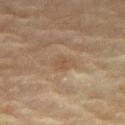Recorded during total-body skin imaging; not selected for excision or biopsy.
Imaged with cross-polarized lighting.
Located on the right thigh.
A female patient, aged 78 to 82.
Measured at roughly 3 mm in maximum diameter.
Cropped from a total-body skin-imaging series; the visible field is about 15 mm.
Automated tile analysis of the lesion measured a lesion area of about 4 mm², a shape eccentricity near 0.75, and a symmetry-axis asymmetry near 0.25. And it measured a border-irregularity rating of about 2.5/10 and internal color variation of about 1.5 on a 0–10 scale. The analysis additionally found a lesion-detection confidence of about 70/100.